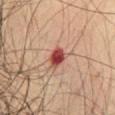The lesion was photographed on a routine skin check and not biopsied; there is no pathology result. Captured under cross-polarized illumination. Automated image analysis of the tile measured a lesion color around L≈39 a*≈27 b*≈24 in CIELAB, about 15 CIELAB-L* units darker than the surrounding skin, and a normalized lesion–skin contrast near 11.5. Longest diameter approximately 2.5 mm. A region of skin cropped from a whole-body photographic capture, roughly 15 mm wide. On the chest. A male patient, aged 73–77.